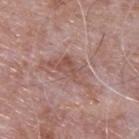This lesion was catalogued during total-body skin photography and was not selected for biopsy. Cropped from a whole-body photographic skin survey; the tile spans about 15 mm. Longest diameter approximately 5.5 mm. A male subject, roughly 65 years of age. An algorithmic analysis of the crop reported a lesion area of about 8 mm², an eccentricity of roughly 0.9, and a symmetry-axis asymmetry near 0.5. It also reported a nevus-likeness score of about 0/100 and lesion-presence confidence of about 95/100. On the upper back.